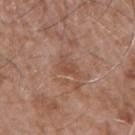Assessment: No biopsy was performed on this lesion — it was imaged during a full skin examination and was not determined to be concerning. Image and clinical context: A male patient, aged 73 to 77. The lesion is located on the arm. The recorded lesion diameter is about 2.5 mm. The tile uses white-light illumination. A lesion tile, about 15 mm wide, cut from a 3D total-body photograph.